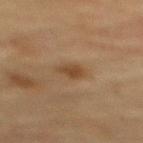Case summary:
* follow-up — no biopsy performed (imaged during a skin exam)
* lesion diameter — about 2.5 mm
* image source — ~15 mm tile from a whole-body skin photo
* patient — female, about 80 years old
* anatomic site — the mid back
* illumination — cross-polarized illumination